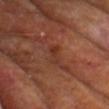Captured during whole-body skin photography for melanoma surveillance; the lesion was not biopsied.
A roughly 15 mm field-of-view crop from a total-body skin photograph.
Captured under cross-polarized illumination.
The patient is a male aged 58 to 62.
Automated tile analysis of the lesion measured an area of roughly 4 mm², an eccentricity of roughly 0.95, and two-axis asymmetry of about 0.25. The analysis additionally found a lesion color around L≈33 a*≈23 b*≈28 in CIELAB and a normalized lesion–skin contrast near 5.5. The software also gave a nevus-likeness score of about 0/100 and lesion-presence confidence of about 85/100.
From the upper back.